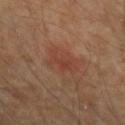workup=no biopsy performed (imaged during a skin exam); site=the arm; image=15 mm crop, total-body photography; patient=male, approximately 55 years of age.